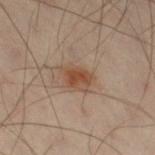{"biopsy_status": "not biopsied; imaged during a skin examination", "image": {"source": "total-body photography crop", "field_of_view_mm": 15}, "patient": {"sex": "male", "age_approx": 50}, "automated_metrics": {"eccentricity": 0.65, "cielab_L": 38, "cielab_a": 16, "cielab_b": 25, "vs_skin_darker_L": 8.0, "vs_skin_contrast_norm": 8.0, "nevus_likeness_0_100": 75, "lesion_detection_confidence_0_100": 100}, "lesion_size": {"long_diameter_mm_approx": 3.5}, "site": "left leg"}